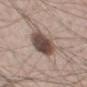Part of a total-body skin-imaging series; this lesion was reviewed on a skin check and was not flagged for biopsy. This image is a 15 mm lesion crop taken from a total-body photograph. From the mid back. The lesion-visualizer software estimated an average lesion color of about L≈47 a*≈15 b*≈21 (CIELAB) and a lesion-to-skin contrast of about 12 (normalized; higher = more distinct). The analysis additionally found a border-irregularity rating of about 1.5/10 and a within-lesion color-variation index near 6.5/10. A male subject, aged 63 to 67. The tile uses white-light illumination.